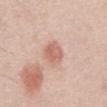The lesion was tiled from a total-body skin photograph and was not biopsied.
Captured under white-light illumination.
A male patient aged approximately 50.
The lesion is located on the abdomen.
Cropped from a whole-body photographic skin survey; the tile spans about 15 mm.